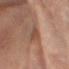Q: What lighting was used for the tile?
A: cross-polarized illumination
Q: What are the patient's age and sex?
A: female, aged 78–82
Q: What did automated image analysis measure?
A: a symmetry-axis asymmetry near 0.25; an average lesion color of about L≈39 a*≈18 b*≈25 (CIELAB) and a normalized border contrast of about 5.5; a color-variation rating of about 1/10 and radial color variation of about 0; a nevus-likeness score of about 0/100 and a lesion-detection confidence of about 80/100
Q: Where on the body is the lesion?
A: the right forearm
Q: What is the imaging modality?
A: ~15 mm crop, total-body skin-cancer survey